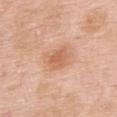Captured during whole-body skin photography for melanoma surveillance; the lesion was not biopsied. On the back. The subject is a female in their 50s. Approximately 4 mm at its widest. This image is a 15 mm lesion crop taken from a total-body photograph. Captured under white-light illumination.